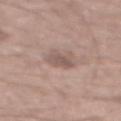Part of a total-body skin-imaging series; this lesion was reviewed on a skin check and was not flagged for biopsy. Imaged with white-light lighting. The lesion is on the mid back. A roughly 15 mm field-of-view crop from a total-body skin photograph. Measured at roughly 3 mm in maximum diameter. A male patient approximately 60 years of age. An algorithmic analysis of the crop reported a mean CIELAB color near L≈54 a*≈16 b*≈21, a lesion–skin lightness drop of about 9, and a lesion-to-skin contrast of about 6 (normalized; higher = more distinct).